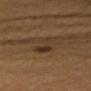{
  "biopsy_status": "not biopsied; imaged during a skin examination",
  "automated_metrics": {
    "vs_skin_darker_L": 6.0
  },
  "patient": {
    "sex": "female",
    "age_approx": 45
  },
  "image": {
    "source": "total-body photography crop",
    "field_of_view_mm": 15
  },
  "site": "left arm",
  "lighting": "cross-polarized",
  "lesion_size": {
    "long_diameter_mm_approx": 3.5
  }
}